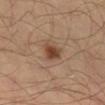biopsy status = no biopsy performed (imaged during a skin exam)
image-analysis metrics = a footprint of about 5 mm² and two-axis asymmetry of about 0.25; an average lesion color of about L≈44 a*≈20 b*≈30 (CIELAB); internal color variation of about 3 on a 0–10 scale and peripheral color asymmetry of about 1; a classifier nevus-likeness of about 95/100 and a detector confidence of about 100 out of 100 that the crop contains a lesion
patient = male, aged 38 to 42
acquisition = 15 mm crop, total-body photography
body site = the right lower leg
size = about 3 mm
lighting = cross-polarized illumination